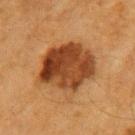location: the right forearm
image source: ~15 mm tile from a whole-body skin photo
size: ≈7 mm
tile lighting: cross-polarized illumination
subject: female, in their mid-50s